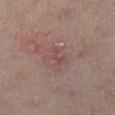Q: Is there a histopathology result?
A: imaged on a skin check; not biopsied
Q: How large is the lesion?
A: ≈2.5 mm
Q: Who is the patient?
A: female, in their mid- to late 50s
Q: How was the tile lit?
A: cross-polarized illumination
Q: What did automated image analysis measure?
A: an average lesion color of about L≈47 a*≈22 b*≈20 (CIELAB) and a lesion-to-skin contrast of about 5 (normalized; higher = more distinct); border irregularity of about 7 on a 0–10 scale, a color-variation rating of about 0/10, and peripheral color asymmetry of about 0
Q: What is the imaging modality?
A: ~15 mm tile from a whole-body skin photo
Q: Where on the body is the lesion?
A: the left lower leg